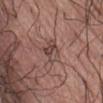<tbp_lesion>
<biopsy_status>not biopsied; imaged during a skin examination</biopsy_status>
<site>front of the torso</site>
<patient>
  <sex>male</sex>
  <age_approx>75</age_approx>
</patient>
<lesion_size>
  <long_diameter_mm_approx>3.0</long_diameter_mm_approx>
</lesion_size>
<image>
  <source>total-body photography crop</source>
  <field_of_view_mm>15</field_of_view_mm>
</image>
</tbp_lesion>